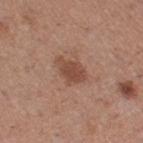The lesion was photographed on a routine skin check and not biopsied; there is no pathology result.
On the right thigh.
A female subject aged around 30.
Imaged with white-light lighting.
A 15 mm close-up tile from a total-body photography series done for melanoma screening.
Measured at roughly 3.5 mm in maximum diameter.